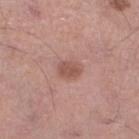{"lesion_size": {"long_diameter_mm_approx": 3.0}, "image": {"source": "total-body photography crop", "field_of_view_mm": 15}, "lighting": "white-light", "patient": {"sex": "male", "age_approx": 55}, "site": "right lower leg", "automated_metrics": {"nevus_likeness_0_100": 65, "lesion_detection_confidence_0_100": 100}}